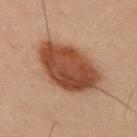Notes:
• biopsy status: no biopsy performed (imaged during a skin exam)
• patient: male, in their mid- to late 50s
• size: about 7 mm
• lighting: cross-polarized illumination
• image: ~15 mm tile from a whole-body skin photo
• body site: the arm